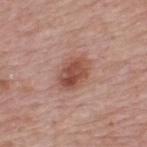{
  "biopsy_status": "not biopsied; imaged during a skin examination",
  "lighting": "white-light",
  "patient": {
    "sex": "male",
    "age_approx": 55
  },
  "image": {
    "source": "total-body photography crop",
    "field_of_view_mm": 15
  },
  "site": "upper back",
  "lesion_size": {
    "long_diameter_mm_approx": 4.0
  }
}